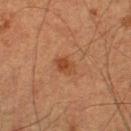This lesion was catalogued during total-body skin photography and was not selected for biopsy.
A 15 mm close-up extracted from a 3D total-body photography capture.
The lesion is located on the leg.
The subject is a male aged around 75.